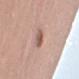The lesion was tiled from a total-body skin photograph and was not biopsied.
The lesion is on the right upper arm.
Measured at roughly 2.5 mm in maximum diameter.
A male subject, aged around 50.
Imaged with white-light lighting.
A 15 mm close-up tile from a total-body photography series done for melanoma screening.
Automated image analysis of the tile measured a lesion area of about 4 mm², a shape eccentricity near 0.7, and a symmetry-axis asymmetry near 0.2. The analysis additionally found a lesion–skin lightness drop of about 11 and a normalized border contrast of about 7.5.